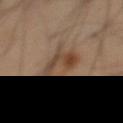This is a cross-polarized tile.
This image is a 15 mm lesion crop taken from a total-body photograph.
The lesion is located on the chest.
The subject is a male aged approximately 55.